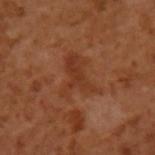No biopsy was performed on this lesion — it was imaged during a full skin examination and was not determined to be concerning. About 5 mm across. A male patient, in their mid-50s. Located on the left upper arm. A 15 mm close-up extracted from a 3D total-body photography capture. Automated tile analysis of the lesion measured a footprint of about 12 mm², an eccentricity of roughly 0.7, and a shape-asymmetry score of about 0.55 (0 = symmetric). The software also gave a mean CIELAB color near L≈37 a*≈25 b*≈33, about 6 CIELAB-L* units darker than the surrounding skin, and a normalized lesion–skin contrast near 6. The software also gave a border-irregularity index near 7/10, internal color variation of about 2.5 on a 0–10 scale, and peripheral color asymmetry of about 1. The analysis additionally found an automated nevus-likeness rating near 0 out of 100 and a lesion-detection confidence of about 100/100.